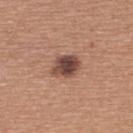| key | value |
|---|---|
| notes | catalogued during a skin exam; not biopsied |
| size | ~3.5 mm (longest diameter) |
| illumination | white-light illumination |
| anatomic site | the back |
| image | 15 mm crop, total-body photography |
| subject | female, roughly 55 years of age |